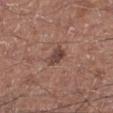A close-up tile cropped from a whole-body skin photograph, about 15 mm across. Located on the left lower leg. Automated image analysis of the tile measured an average lesion color of about L≈43 a*≈19 b*≈23 (CIELAB), about 10 CIELAB-L* units darker than the surrounding skin, and a lesion-to-skin contrast of about 8 (normalized; higher = more distinct). It also reported border irregularity of about 2 on a 0–10 scale and a peripheral color-asymmetry measure near 1. And it measured a classifier nevus-likeness of about 35/100 and lesion-presence confidence of about 100/100. The tile uses white-light illumination. The subject is a male aged 53–57. Measured at roughly 2.5 mm in maximum diameter.